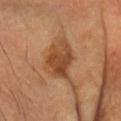The lesion was tiled from a total-body skin photograph and was not biopsied. Captured under cross-polarized illumination. The lesion is on the head or neck. A male subject, in their mid-60s. The total-body-photography lesion software estimated a mean CIELAB color near L≈43 a*≈23 b*≈35, roughly 11 lightness units darker than nearby skin, and a normalized lesion–skin contrast near 9. And it measured border irregularity of about 4.5 on a 0–10 scale, internal color variation of about 3 on a 0–10 scale, and peripheral color asymmetry of about 1. It also reported a nevus-likeness score of about 20/100 and a lesion-detection confidence of about 100/100. This image is a 15 mm lesion crop taken from a total-body photograph.